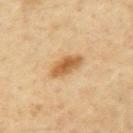Q: Was a biopsy performed?
A: catalogued during a skin exam; not biopsied
Q: Who is the patient?
A: male, roughly 65 years of age
Q: What kind of image is this?
A: 15 mm crop, total-body photography
Q: Where on the body is the lesion?
A: the left upper arm
Q: What did automated image analysis measure?
A: a mean CIELAB color near L≈59 a*≈20 b*≈41, a lesion–skin lightness drop of about 13, and a lesion-to-skin contrast of about 8.5 (normalized; higher = more distinct); a nevus-likeness score of about 95/100 and lesion-presence confidence of about 100/100
Q: How large is the lesion?
A: about 4 mm